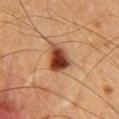workup — imaged on a skin check; not biopsied | location — the chest | imaging modality — ~15 mm crop, total-body skin-cancer survey | patient — male, roughly 50 years of age | size — ~3.5 mm (longest diameter) | tile lighting — cross-polarized illumination.